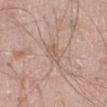A male subject approximately 60 years of age. A 15 mm close-up extracted from a 3D total-body photography capture. Located on the right lower leg.